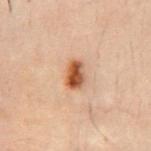Assessment:
Imaged during a routine full-body skin examination; the lesion was not biopsied and no histopathology is available.
Background:
Longest diameter approximately 3 mm. A male subject, about 30 years old. Captured under cross-polarized illumination. Located on the chest. A 15 mm close-up extracted from a 3D total-body photography capture.